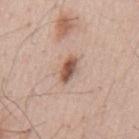site — the mid back | patient — male, aged 53–57 | image source — total-body-photography crop, ~15 mm field of view.